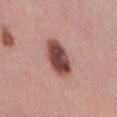follow-up: no biopsy performed (imaged during a skin exam)
acquisition: total-body-photography crop, ~15 mm field of view
site: the lower back
subject: female, in their 30s
lesion diameter: about 4.5 mm
automated lesion analysis: a lesion area of about 11 mm² and an outline eccentricity of about 0.8 (0 = round, 1 = elongated); a within-lesion color-variation index near 5.5/10; a classifier nevus-likeness of about 95/100 and a detector confidence of about 100 out of 100 that the crop contains a lesion